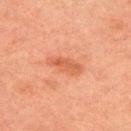Impression: Recorded during total-body skin imaging; not selected for excision or biopsy. Background: The lesion is on the upper back. Measured at roughly 4 mm in maximum diameter. Automated image analysis of the tile measured a shape eccentricity near 0.9 and two-axis asymmetry of about 0.25. The subject is a male aged approximately 50. Imaged with cross-polarized lighting. A 15 mm close-up extracted from a 3D total-body photography capture.